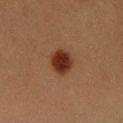notes: catalogued during a skin exam; not biopsied | imaging modality: total-body-photography crop, ~15 mm field of view | subject: male, roughly 40 years of age | location: the left forearm | illumination: cross-polarized.